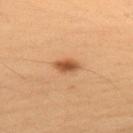Q: Was a biopsy performed?
A: imaged on a skin check; not biopsied
Q: What is the anatomic site?
A: the upper back
Q: What is the imaging modality?
A: 15 mm crop, total-body photography
Q: Lesion size?
A: ≈3 mm
Q: Illumination type?
A: cross-polarized
Q: What are the patient's age and sex?
A: male, roughly 55 years of age